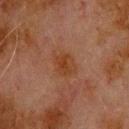This lesion was catalogued during total-body skin photography and was not selected for biopsy.
Located on the upper back.
The tile uses cross-polarized illumination.
A close-up tile cropped from a whole-body skin photograph, about 15 mm across.
A male subject, aged 78–82.
Automated image analysis of the tile measured an average lesion color of about L≈31 a*≈19 b*≈28 (CIELAB) and roughly 5 lightness units darker than nearby skin. The analysis additionally found a within-lesion color-variation index near 3/10 and a peripheral color-asymmetry measure near 1. The analysis additionally found a nevus-likeness score of about 25/100 and a lesion-detection confidence of about 100/100.